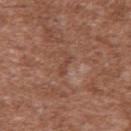{
  "biopsy_status": "not biopsied; imaged during a skin examination",
  "image": {
    "source": "total-body photography crop",
    "field_of_view_mm": 15
  },
  "lesion_size": {
    "long_diameter_mm_approx": 2.5
  },
  "patient": {
    "sex": "male",
    "age_approx": 45
  },
  "lighting": "white-light",
  "site": "upper back"
}